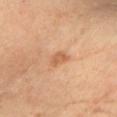Recorded during total-body skin imaging; not selected for excision or biopsy.
A female subject, aged approximately 60.
Imaged with cross-polarized lighting.
A roughly 15 mm field-of-view crop from a total-body skin photograph.
The total-body-photography lesion software estimated an average lesion color of about L≈60 a*≈24 b*≈38 (CIELAB), a lesion–skin lightness drop of about 9, and a normalized border contrast of about 6.5. It also reported border irregularity of about 2.5 on a 0–10 scale, a within-lesion color-variation index near 2/10, and radial color variation of about 0.5. It also reported a classifier nevus-likeness of about 15/100.
On the chest.